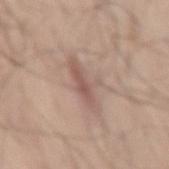  biopsy_status: not biopsied; imaged during a skin examination
  patient:
    sex: male
    age_approx: 70
  image:
    source: total-body photography crop
    field_of_view_mm: 15
  site: right thigh
  lighting: white-light
  lesion_size:
    long_diameter_mm_approx: 5.5